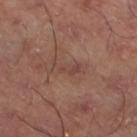Assessment:
The lesion was photographed on a routine skin check and not biopsied; there is no pathology result.
Context:
The tile uses cross-polarized illumination. Measured at roughly 3.5 mm in maximum diameter. A region of skin cropped from a whole-body photographic capture, roughly 15 mm wide. Located on the left thigh. Automated image analysis of the tile measured internal color variation of about 0.5 on a 0–10 scale and peripheral color asymmetry of about 0.